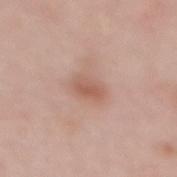Captured during whole-body skin photography for melanoma surveillance; the lesion was not biopsied. On the mid back. This image is a 15 mm lesion crop taken from a total-body photograph. A female patient, aged 48 to 52.